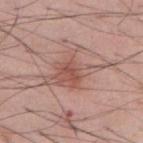{
  "biopsy_status": "not biopsied; imaged during a skin examination",
  "site": "chest",
  "image": {
    "source": "total-body photography crop",
    "field_of_view_mm": 15
  },
  "patient": {
    "sex": "male",
    "age_approx": 55
  }
}